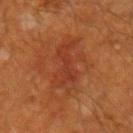Case summary:
– notes · no biopsy performed (imaged during a skin exam)
– patient · male, approximately 60 years of age
– site · the right forearm
– acquisition · ~15 mm crop, total-body skin-cancer survey
– lesion diameter · ≈5.5 mm
– tile lighting · cross-polarized illumination
– automated lesion analysis · an area of roughly 11 mm², an eccentricity of roughly 0.9, and a symmetry-axis asymmetry near 0.4; a mean CIELAB color near L≈36 a*≈28 b*≈33, roughly 6 lightness units darker than nearby skin, and a normalized lesion–skin contrast near 5.5